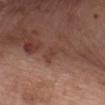Assessment:
Captured during whole-body skin photography for melanoma surveillance; the lesion was not biopsied.
Image and clinical context:
Imaged with white-light lighting. Approximately 3 mm at its widest. A lesion tile, about 15 mm wide, cut from a 3D total-body photograph. A female patient roughly 75 years of age. Located on the chest.